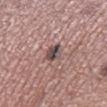Clinical impression:
The lesion was photographed on a routine skin check and not biopsied; there is no pathology result.
Context:
The tile uses white-light illumination. From the right lower leg. A female patient aged approximately 40. Automated image analysis of the tile measured a lesion color around L≈45 a*≈15 b*≈16 in CIELAB and a lesion–skin lightness drop of about 14. A 15 mm crop from a total-body photograph taken for skin-cancer surveillance. Approximately 2.5 mm at its widest.